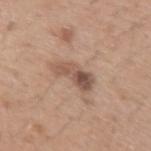<lesion>
<biopsy_status>not biopsied; imaged during a skin examination</biopsy_status>
<lighting>white-light</lighting>
<image>
  <source>total-body photography crop</source>
  <field_of_view_mm>15</field_of_view_mm>
</image>
<automated_metrics>
  <area_mm2_approx>8.0</area_mm2_approx>
  <shape_asymmetry>0.35</shape_asymmetry>
  <cielab_L>53</cielab_L>
  <cielab_a>18</cielab_a>
  <cielab_b>27</cielab_b>
  <vs_skin_darker_L>11.0</vs_skin_darker_L>
  <vs_skin_contrast_norm>7.5</vs_skin_contrast_norm>
  <nevus_likeness_0_100>15</nevus_likeness_0_100>
</automated_metrics>
<patient>
  <sex>male</sex>
  <age_approx>40</age_approx>
</patient>
<site>left upper arm</site>
</lesion>